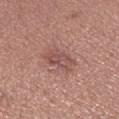Impression: Captured during whole-body skin photography for melanoma surveillance; the lesion was not biopsied. Context: The recorded lesion diameter is about 4 mm. A female patient aged 33 to 37. The lesion is on the right lower leg. Imaged with white-light lighting. A region of skin cropped from a whole-body photographic capture, roughly 15 mm wide.